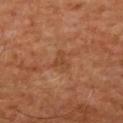Recorded during total-body skin imaging; not selected for excision or biopsy. The tile uses cross-polarized illumination. A male subject, aged 58–62. A lesion tile, about 15 mm wide, cut from a 3D total-body photograph. The recorded lesion diameter is about 2.5 mm. The lesion is on the chest.